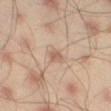Impression:
Recorded during total-body skin imaging; not selected for excision or biopsy.
Clinical summary:
A 15 mm close-up extracted from a 3D total-body photography capture. The lesion is on the left thigh. About 3 mm across. A male subject, in their mid- to late 40s. Automated tile analysis of the lesion measured a lesion color around L≈61 a*≈16 b*≈28 in CIELAB, about 7 CIELAB-L* units darker than the surrounding skin, and a normalized lesion–skin contrast near 4.5.